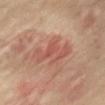Q: Was this lesion biopsied?
A: total-body-photography surveillance lesion; no biopsy